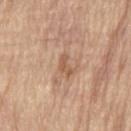Case summary:
– lesion diameter: about 2.5 mm
– patient: male, in their 70s
– tile lighting: white-light
– acquisition: ~15 mm crop, total-body skin-cancer survey
– site: the lower back